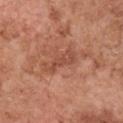biopsy_status: not biopsied; imaged during a skin examination
lesion_size:
  long_diameter_mm_approx: 4.5
image:
  source: total-body photography crop
  field_of_view_mm: 15
patient:
  sex: female
  age_approx: 50
lighting: white-light
site: chest